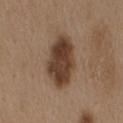| field | value |
|---|---|
| follow-up | total-body-photography surveillance lesion; no biopsy |
| diameter | ≈6.5 mm |
| site | the mid back |
| tile lighting | white-light illumination |
| imaging modality | ~15 mm crop, total-body skin-cancer survey |
| TBP lesion metrics | border irregularity of about 2 on a 0–10 scale, internal color variation of about 4.5 on a 0–10 scale, and radial color variation of about 1.5 |
| subject | female, roughly 40 years of age |